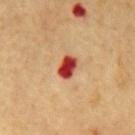The subject is a male in their mid- to late 60s. On the mid back. This is a cross-polarized tile. Longest diameter approximately 3 mm. A 15 mm crop from a total-body photograph taken for skin-cancer surveillance.